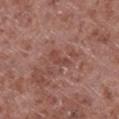<lesion>
<image>
  <source>total-body photography crop</source>
  <field_of_view_mm>15</field_of_view_mm>
</image>
<site>left lower leg</site>
<patient>
  <sex>male</sex>
  <age_approx>55</age_approx>
</patient>
<lesion_size>
  <long_diameter_mm_approx>3.0</long_diameter_mm_approx>
</lesion_size>
</lesion>